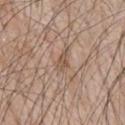follow-up=no biopsy performed (imaged during a skin exam); tile lighting=white-light; body site=the chest; acquisition=15 mm crop, total-body photography; patient=male, aged around 65; lesion diameter=~2.5 mm (longest diameter).